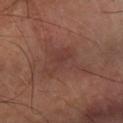<tbp_lesion>
  <biopsy_status>not biopsied; imaged during a skin examination</biopsy_status>
  <site>left forearm</site>
  <image>
    <source>total-body photography crop</source>
    <field_of_view_mm>15</field_of_view_mm>
  </image>
  <patient>
    <age_approx>65</age_approx>
  </patient>
</tbp_lesion>